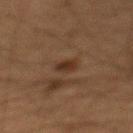Q: Was this lesion biopsied?
A: no biopsy performed (imaged during a skin exam)
Q: What are the patient's age and sex?
A: male, aged around 65
Q: Lesion location?
A: the mid back
Q: How was this image acquired?
A: total-body-photography crop, ~15 mm field of view